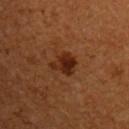| key | value |
|---|---|
| follow-up | no biopsy performed (imaged during a skin exam) |